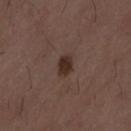The lesion was tiled from a total-body skin photograph and was not biopsied.